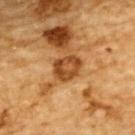• workup — total-body-photography surveillance lesion; no biopsy
• lighting — cross-polarized illumination
• subject — male, approximately 85 years of age or older
• location — the upper back
• acquisition — total-body-photography crop, ~15 mm field of view
• size — about 3.5 mm